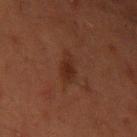– workup — catalogued during a skin exam; not biopsied
– image — total-body-photography crop, ~15 mm field of view
– size — ~3 mm (longest diameter)
– anatomic site — the left upper arm
– illumination — cross-polarized illumination
– patient — male, approximately 45 years of age